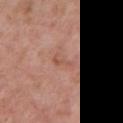The lesion is located on the chest. Longest diameter approximately 3 mm. Imaged with white-light lighting. A 15 mm close-up extracted from a 3D total-body photography capture. A female subject approximately 40 years of age.